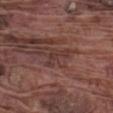The lesion was tiled from a total-body skin photograph and was not biopsied. A 15 mm close-up tile from a total-body photography series done for melanoma screening. The subject is a male aged 73–77. On the arm.